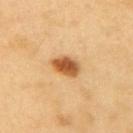lesion_size:
  long_diameter_mm_approx: 3.5
patient:
  sex: male
  age_approx: 60
automated_metrics:
  area_mm2_approx: 6.5
  eccentricity: 0.7
  vs_skin_darker_L: 16.0
  vs_skin_contrast_norm: 10.5
  border_irregularity_0_10: 1.5
  color_variation_0_10: 5.0
  nevus_likeness_0_100: 100
lighting: cross-polarized
image:
  source: total-body photography crop
  field_of_view_mm: 15
site: right upper arm